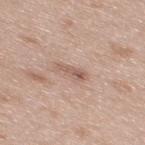Assessment: Recorded during total-body skin imaging; not selected for excision or biopsy. Clinical summary: From the upper back. Captured under white-light illumination. About 3 mm across. The patient is a female aged approximately 40. Cropped from a total-body skin-imaging series; the visible field is about 15 mm.